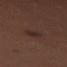Q: Was a biopsy performed?
A: imaged on a skin check; not biopsied
Q: Lesion size?
A: ~3 mm (longest diameter)
Q: How was this image acquired?
A: 15 mm crop, total-body photography
Q: Lesion location?
A: the right thigh
Q: Patient demographics?
A: female, aged around 50
Q: What lighting was used for the tile?
A: cross-polarized